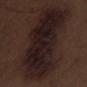Notes:
- biopsy status · total-body-photography surveillance lesion; no biopsy